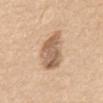Notes:
– biopsy status · no biopsy performed (imaged during a skin exam)
– image · total-body-photography crop, ~15 mm field of view
– lesion size · ≈5.5 mm
– site · the abdomen
– tile lighting · white-light
– patient · female, roughly 70 years of age